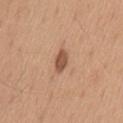The lesion was tiled from a total-body skin photograph and was not biopsied.
Located on the lower back.
Longest diameter approximately 3 mm.
A male patient aged 33–37.
A 15 mm close-up extracted from a 3D total-body photography capture.